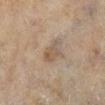Notes:
– follow-up: total-body-photography surveillance lesion; no biopsy
– illumination: cross-polarized illumination
– patient: female, approximately 60 years of age
– location: the left lower leg
– imaging modality: total-body-photography crop, ~15 mm field of view
– lesion size: about 3.5 mm